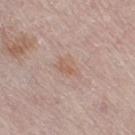No biopsy was performed on this lesion — it was imaged during a full skin examination and was not determined to be concerning.
This image is a 15 mm lesion crop taken from a total-body photograph.
A female subject aged 63 to 67.
Measured at roughly 3.5 mm in maximum diameter.
The lesion is on the leg.
Imaged with white-light lighting.
The lesion-visualizer software estimated a lesion area of about 5.5 mm², an eccentricity of roughly 0.6, and a shape-asymmetry score of about 0.35 (0 = symmetric). It also reported an average lesion color of about L≈60 a*≈17 b*≈26 (CIELAB), a lesion–skin lightness drop of about 6, and a lesion-to-skin contrast of about 5 (normalized; higher = more distinct). And it measured an automated nevus-likeness rating near 0 out of 100 and a detector confidence of about 100 out of 100 that the crop contains a lesion.